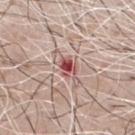biopsy_status: not biopsied; imaged during a skin examination
lesion_size:
  long_diameter_mm_approx: 5.0
patient:
  sex: male
  age_approx: 70
image:
  source: total-body photography crop
  field_of_view_mm: 15
automated_metrics:
  cielab_L: 56
  cielab_a: 24
  cielab_b: 22
  vs_skin_darker_L: 13.0
  vs_skin_contrast_norm: 8.5
  nevus_likeness_0_100: 0
  lesion_detection_confidence_0_100: 100
site: chest